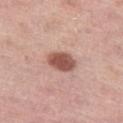Recorded during total-body skin imaging; not selected for excision or biopsy. The lesion is located on the left thigh. Measured at roughly 3.5 mm in maximum diameter. A female subject, aged around 45. A 15 mm close-up extracted from a 3D total-body photography capture.